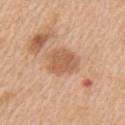Recorded during total-body skin imaging; not selected for excision or biopsy. A male subject, approximately 60 years of age. About 4 mm across. On the left upper arm. A lesion tile, about 15 mm wide, cut from a 3D total-body photograph. Automated image analysis of the tile measured a lesion area of about 10 mm², an eccentricity of roughly 0.5, and two-axis asymmetry of about 0.15. The software also gave a mean CIELAB color near L≈59 a*≈22 b*≈34 and roughly 10 lightness units darker than nearby skin. The analysis additionally found border irregularity of about 2 on a 0–10 scale. This is a white-light tile.